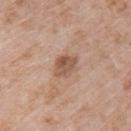The lesion was photographed on a routine skin check and not biopsied; there is no pathology result.
Automated tile analysis of the lesion measured an area of roughly 5.5 mm² and two-axis asymmetry of about 0.2. The software also gave a border-irregularity index near 2/10, a color-variation rating of about 5/10, and a peripheral color-asymmetry measure near 1.5.
This is a white-light tile.
A 15 mm close-up extracted from a 3D total-body photography capture.
The lesion is located on the left upper arm.
Longest diameter approximately 3 mm.
A female subject, in their 70s.